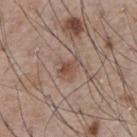Captured during whole-body skin photography for melanoma surveillance; the lesion was not biopsied.
A region of skin cropped from a whole-body photographic capture, roughly 15 mm wide.
Longest diameter approximately 3 mm.
From the chest.
This is a white-light tile.
A male subject, aged around 75.
Automated image analysis of the tile measured a lesion color around L≈50 a*≈17 b*≈26 in CIELAB and a normalized lesion–skin contrast near 6.5. The analysis additionally found internal color variation of about 3.5 on a 0–10 scale and radial color variation of about 1.5.